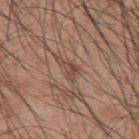Longest diameter approximately 3 mm.
This is a white-light tile.
A close-up tile cropped from a whole-body skin photograph, about 15 mm across.
A male patient aged approximately 45.
On the back.